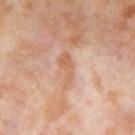Q: Was this lesion biopsied?
A: catalogued during a skin exam; not biopsied
Q: How large is the lesion?
A: ~4 mm (longest diameter)
Q: Patient demographics?
A: female, aged approximately 55
Q: Illumination type?
A: cross-polarized illumination
Q: Where on the body is the lesion?
A: the leg
Q: Automated lesion metrics?
A: a shape eccentricity near 0.95; border irregularity of about 5 on a 0–10 scale and radial color variation of about 0.5
Q: What is the imaging modality?
A: ~15 mm crop, total-body skin-cancer survey